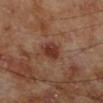<case>
<biopsy_status>not biopsied; imaged during a skin examination</biopsy_status>
<lighting>cross-polarized</lighting>
<site>leg</site>
<image>
  <source>total-body photography crop</source>
  <field_of_view_mm>15</field_of_view_mm>
</image>
<patient>
  <sex>male</sex>
  <age_approx>70</age_approx>
</patient>
<automated_metrics>
  <area_mm2_approx>6.0</area_mm2_approx>
  <shape_asymmetry>0.2</shape_asymmetry>
  <border_irregularity_0_10>2.0</border_irregularity_0_10>
  <color_variation_0_10>3.0</color_variation_0_10>
  <peripheral_color_asymmetry>1.0</peripheral_color_asymmetry>
</automated_metrics>
<lesion_size>
  <long_diameter_mm_approx>3.0</long_diameter_mm_approx>
</lesion_size>
</case>